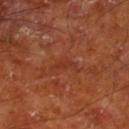Longest diameter approximately 2.5 mm. The tile uses cross-polarized illumination. Automated image analysis of the tile measured an eccentricity of roughly 0.9 and two-axis asymmetry of about 0.25. The software also gave a within-lesion color-variation index near 0/10 and a peripheral color-asymmetry measure near 0. And it measured a nevus-likeness score of about 0/100. From the right lower leg. A male subject, aged 63–67. This image is a 15 mm lesion crop taken from a total-body photograph.